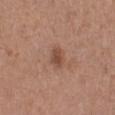Case summary:
* biopsy status — no biopsy performed (imaged during a skin exam)
* subject — female, approximately 30 years of age
* tile lighting — white-light illumination
* image source — ~15 mm tile from a whole-body skin photo
* anatomic site — the left lower leg